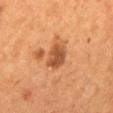Captured during whole-body skin photography for melanoma surveillance; the lesion was not biopsied. A 15 mm crop from a total-body photograph taken for skin-cancer surveillance. The lesion is on the mid back. A male patient, about 55 years old. The recorded lesion diameter is about 3 mm. The tile uses cross-polarized illumination.